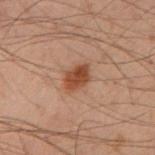notes — total-body-photography surveillance lesion; no biopsy | location — the arm | lesion diameter — about 3 mm | subject — male, approximately 40 years of age | acquisition — ~15 mm tile from a whole-body skin photo | lighting — cross-polarized.